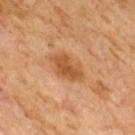Impression: Recorded during total-body skin imaging; not selected for excision or biopsy. Clinical summary: A 15 mm crop from a total-body photograph taken for skin-cancer surveillance. An algorithmic analysis of the crop reported an average lesion color of about L≈53 a*≈24 b*≈40 (CIELAB), about 10 CIELAB-L* units darker than the surrounding skin, and a normalized border contrast of about 8. Longest diameter approximately 4.5 mm. A male patient roughly 65 years of age. The tile uses cross-polarized illumination.